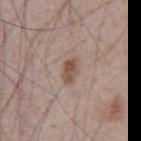notes — imaged on a skin check; not biopsied
image source — total-body-photography crop, ~15 mm field of view
location — the chest
subject — male, about 65 years old